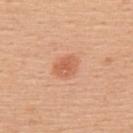biopsy status = no biopsy performed (imaged during a skin exam).